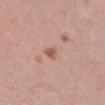workup: total-body-photography surveillance lesion; no biopsy | anatomic site: the right thigh | image: ~15 mm tile from a whole-body skin photo | subject: female, aged approximately 40.